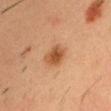Q: Was a biopsy performed?
A: imaged on a skin check; not biopsied
Q: What are the patient's age and sex?
A: male, approximately 35 years of age
Q: Where on the body is the lesion?
A: the head or neck
Q: Lesion size?
A: ≈3 mm
Q: How was this image acquired?
A: ~15 mm tile from a whole-body skin photo
Q: How was the tile lit?
A: cross-polarized illumination